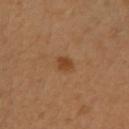No biopsy was performed on this lesion — it was imaged during a full skin examination and was not determined to be concerning. The lesion is located on the right upper arm. The patient is a female aged 28–32. A close-up tile cropped from a whole-body skin photograph, about 15 mm across.